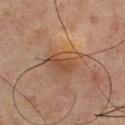An algorithmic analysis of the crop reported an outline eccentricity of about 0.7 (0 = round, 1 = elongated) and a shape-asymmetry score of about 0.4 (0 = symmetric). The patient is a male roughly 65 years of age. The lesion is on the upper back. Cropped from a total-body skin-imaging series; the visible field is about 15 mm.